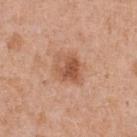Assessment: Imaged during a routine full-body skin examination; the lesion was not biopsied and no histopathology is available. Clinical summary: About 3 mm across. Captured under white-light illumination. The lesion-visualizer software estimated a lesion color around L≈54 a*≈24 b*≈33 in CIELAB, roughly 11 lightness units darker than nearby skin, and a normalized lesion–skin contrast near 7.5. The analysis additionally found a color-variation rating of about 6/10 and a peripheral color-asymmetry measure near 2. And it measured a nevus-likeness score of about 70/100 and a detector confidence of about 100 out of 100 that the crop contains a lesion. This image is a 15 mm lesion crop taken from a total-body photograph. The lesion is located on the chest. A male patient about 55 years old.